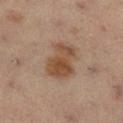Clinical impression:
The lesion was tiled from a total-body skin photograph and was not biopsied.
Acquisition and patient details:
On the right lower leg. About 4.5 mm across. Automated tile analysis of the lesion measured a lesion area of about 14 mm², a shape eccentricity near 0.65, and a symmetry-axis asymmetry near 0.2. It also reported an average lesion color of about L≈47 a*≈17 b*≈29 (CIELAB) and roughly 10 lightness units darker than nearby skin. And it measured a border-irregularity index near 2.5/10, a within-lesion color-variation index near 5/10, and peripheral color asymmetry of about 1.5. It also reported a classifier nevus-likeness of about 85/100 and a lesion-detection confidence of about 100/100. A 15 mm close-up tile from a total-body photography series done for melanoma screening. Imaged with cross-polarized lighting. A male subject in their mid-50s.